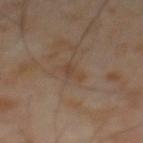This lesion was catalogued during total-body skin photography and was not selected for biopsy. The subject is a male in their mid-60s. Automated image analysis of the tile measured a border-irregularity rating of about 5.5/10, internal color variation of about 0 on a 0–10 scale, and radial color variation of about 0. This is a cross-polarized tile. Approximately 2.5 mm at its widest. A 15 mm close-up tile from a total-body photography series done for melanoma screening.